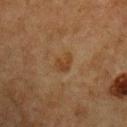Q: Is there a histopathology result?
A: catalogued during a skin exam; not biopsied
Q: Who is the patient?
A: male, in their mid- to late 70s
Q: What is the imaging modality?
A: total-body-photography crop, ~15 mm field of view
Q: Lesion location?
A: the front of the torso